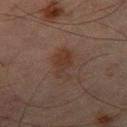Assessment:
No biopsy was performed on this lesion — it was imaged during a full skin examination and was not determined to be concerning.
Background:
From the left thigh. Longest diameter approximately 4 mm. Imaged with cross-polarized lighting. A male patient, aged around 65. A 15 mm close-up extracted from a 3D total-body photography capture. The lesion-visualizer software estimated a lesion area of about 7 mm². The analysis additionally found an average lesion color of about L≈26 a*≈14 b*≈20 (CIELAB), roughly 5 lightness units darker than nearby skin, and a normalized border contrast of about 6.5. The software also gave a classifier nevus-likeness of about 70/100 and lesion-presence confidence of about 100/100.